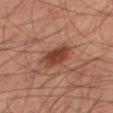notes=catalogued during a skin exam; not biopsied
lesion size=~4 mm (longest diameter)
tile lighting=cross-polarized illumination
image=15 mm crop, total-body photography
body site=the right thigh
patient=male, aged 58–62